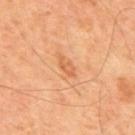The lesion was photographed on a routine skin check and not biopsied; there is no pathology result. A male subject approximately 65 years of age. On the mid back. A 15 mm crop from a total-body photograph taken for skin-cancer surveillance.